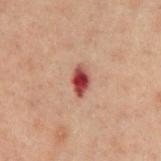{"image": {"source": "total-body photography crop", "field_of_view_mm": 15}, "site": "chest", "patient": {"sex": "male", "age_approx": 60}}